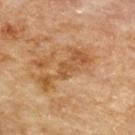The lesion was tiled from a total-body skin photograph and was not biopsied.
On the upper back.
A male patient in their mid- to late 80s or older.
Approximately 7 mm at its widest.
A lesion tile, about 15 mm wide, cut from a 3D total-body photograph.